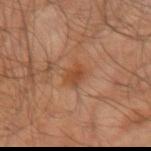• biopsy status: total-body-photography surveillance lesion; no biopsy